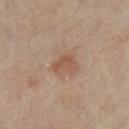No biopsy was performed on this lesion — it was imaged during a full skin examination and was not determined to be concerning. An algorithmic analysis of the crop reported roughly 8 lightness units darker than nearby skin and a normalized lesion–skin contrast near 6. The software also gave border irregularity of about 3 on a 0–10 scale, a color-variation rating of about 2/10, and peripheral color asymmetry of about 0.5. The tile uses cross-polarized illumination. The lesion's longest dimension is about 3 mm. From the left lower leg. The patient is a female aged approximately 65. A close-up tile cropped from a whole-body skin photograph, about 15 mm across.